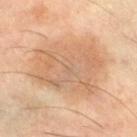workup: total-body-photography surveillance lesion; no biopsy | subject: male, aged 58–62 | location: the right thigh | acquisition: total-body-photography crop, ~15 mm field of view | illumination: cross-polarized | size: ≈9 mm.